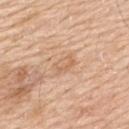Imaged during a routine full-body skin examination; the lesion was not biopsied and no histopathology is available.
The recorded lesion diameter is about 3 mm.
The patient is a male aged approximately 60.
A roughly 15 mm field-of-view crop from a total-body skin photograph.
Imaged with white-light lighting.
On the upper back.